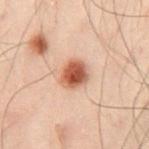Case summary:
- biopsy status — no biopsy performed (imaged during a skin exam)
- tile lighting — cross-polarized illumination
- location — the left leg
- image source — total-body-photography crop, ~15 mm field of view
- subject — male, aged approximately 50
- lesion size — ≈3 mm
- TBP lesion metrics — a lesion area of about 7.5 mm², an outline eccentricity of about 0.35 (0 = round, 1 = elongated), and a symmetry-axis asymmetry near 0.2; a lesion color around L≈46 a*≈22 b*≈28 in CIELAB, roughly 14 lightness units darker than nearby skin, and a normalized lesion–skin contrast near 11; lesion-presence confidence of about 100/100